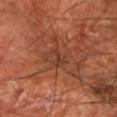follow-up = imaged on a skin check; not biopsied | diameter = ≈2.5 mm | location = the arm | patient = male, aged 68 to 72 | acquisition = ~15 mm tile from a whole-body skin photo.